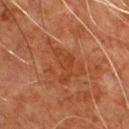| field | value |
|---|---|
| biopsy status | total-body-photography surveillance lesion; no biopsy |
| subject | male, roughly 80 years of age |
| illumination | cross-polarized illumination |
| image | 15 mm crop, total-body photography |
| site | the chest |
| lesion size | ≈6 mm |